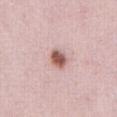* biopsy status: total-body-photography surveillance lesion; no biopsy